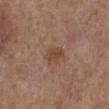The lesion was photographed on a routine skin check and not biopsied; there is no pathology result. A close-up tile cropped from a whole-body skin photograph, about 15 mm across. A female subject, approximately 65 years of age. Imaged with white-light lighting. From the left lower leg. The lesion's longest dimension is about 3 mm.